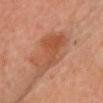Recorded during total-body skin imaging; not selected for excision or biopsy.
The lesion is on the chest.
This is a cross-polarized tile.
The lesion's longest dimension is about 8 mm.
The patient is a male in their 60s.
Cropped from a whole-body photographic skin survey; the tile spans about 15 mm.